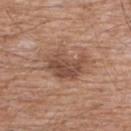No biopsy was performed on this lesion — it was imaged during a full skin examination and was not determined to be concerning. An algorithmic analysis of the crop reported an outline eccentricity of about 0.75 (0 = round, 1 = elongated). And it measured a border-irregularity rating of about 4.5/10, internal color variation of about 4 on a 0–10 scale, and a peripheral color-asymmetry measure near 1.5. And it measured an automated nevus-likeness rating near 35 out of 100 and lesion-presence confidence of about 100/100. The lesion's longest dimension is about 5 mm. A 15 mm close-up tile from a total-body photography series done for melanoma screening. The tile uses white-light illumination. A male subject roughly 60 years of age. From the chest.